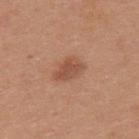The lesion was photographed on a routine skin check and not biopsied; there is no pathology result.
The tile uses white-light illumination.
The lesion is located on the upper back.
Cropped from a whole-body photographic skin survey; the tile spans about 15 mm.
The subject is a male approximately 30 years of age.
The total-body-photography lesion software estimated an area of roughly 6.5 mm² and an outline eccentricity of about 0.8 (0 = round, 1 = elongated).
About 3.5 mm across.